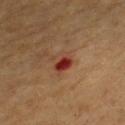The lesion was tiled from a total-body skin photograph and was not biopsied. The tile uses cross-polarized illumination. The lesion is located on the chest. Cropped from a whole-body photographic skin survey; the tile spans about 15 mm. A male patient, about 55 years old. Longest diameter approximately 2.5 mm. Automated tile analysis of the lesion measured a lesion area of about 5 mm², a shape eccentricity near 0.55, and a symmetry-axis asymmetry near 0.25. And it measured a lesion color around L≈32 a*≈26 b*≈27 in CIELAB and a lesion-to-skin contrast of about 9.5 (normalized; higher = more distinct). And it measured a classifier nevus-likeness of about 0/100 and a lesion-detection confidence of about 100/100.